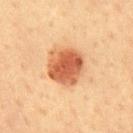Imaged during a routine full-body skin examination; the lesion was not biopsied and no histopathology is available.
A male patient, aged 58 to 62.
Automated tile analysis of the lesion measured a footprint of about 15 mm², an outline eccentricity of about 0.3 (0 = round, 1 = elongated), and two-axis asymmetry of about 0.15. And it measured a lesion color around L≈53 a*≈26 b*≈36 in CIELAB, about 15 CIELAB-L* units darker than the surrounding skin, and a normalized lesion–skin contrast near 10. The software also gave border irregularity of about 1.5 on a 0–10 scale, a color-variation rating of about 5/10, and a peripheral color-asymmetry measure near 1.5.
This image is a 15 mm lesion crop taken from a total-body photograph.
Longest diameter approximately 4.5 mm.
Located on the chest.